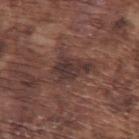Q: Was a biopsy performed?
A: imaged on a skin check; not biopsied
Q: What is the anatomic site?
A: the left upper arm
Q: Who is the patient?
A: male, aged around 75
Q: How large is the lesion?
A: about 5 mm
Q: Automated lesion metrics?
A: roughly 8 lightness units darker than nearby skin and a lesion-to-skin contrast of about 8.5 (normalized; higher = more distinct)
Q: What kind of image is this?
A: ~15 mm tile from a whole-body skin photo
Q: What lighting was used for the tile?
A: white-light illumination